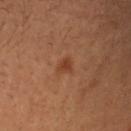{"automated_metrics": {"area_mm2_approx": 3.0, "eccentricity": 0.85, "shape_asymmetry": 0.3, "nevus_likeness_0_100": 40, "lesion_detection_confidence_0_100": 100}, "lesion_size": {"long_diameter_mm_approx": 2.5}, "site": "head or neck", "image": {"source": "total-body photography crop", "field_of_view_mm": 15}, "lighting": "cross-polarized", "patient": {"sex": "male", "age_approx": 30}}